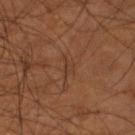Part of a total-body skin-imaging series; this lesion was reviewed on a skin check and was not flagged for biopsy. Located on the left lower leg. Automated tile analysis of the lesion measured a lesion–skin lightness drop of about 5 and a normalized border contrast of about 4.5. The software also gave a border-irregularity index near 5.5/10, a color-variation rating of about 0.5/10, and a peripheral color-asymmetry measure near 0.5. Measured at roughly 2.5 mm in maximum diameter. Imaged with cross-polarized lighting. A male subject about 55 years old. A 15 mm crop from a total-body photograph taken for skin-cancer surveillance.